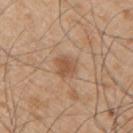This lesion was catalogued during total-body skin photography and was not selected for biopsy. A male patient, aged around 50. Located on the upper back. Cropped from a whole-body photographic skin survey; the tile spans about 15 mm. Longest diameter approximately 3 mm. An algorithmic analysis of the crop reported a lesion area of about 5.5 mm², an outline eccentricity of about 0.5 (0 = round, 1 = elongated), and a shape-asymmetry score of about 0.2 (0 = symmetric). It also reported a mean CIELAB color near L≈54 a*≈19 b*≈33, about 9 CIELAB-L* units darker than the surrounding skin, and a normalized border contrast of about 6.5. Captured under white-light illumination.